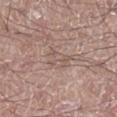biopsy_status: not biopsied; imaged during a skin examination
image:
  source: total-body photography crop
  field_of_view_mm: 15
site: leg
patient:
  sex: male
  age_approx: 60